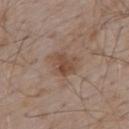The lesion was photographed on a routine skin check and not biopsied; there is no pathology result.